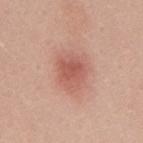workup=no biopsy performed (imaged during a skin exam)
imaging modality=~15 mm crop, total-body skin-cancer survey
location=the back
subject=male, aged approximately 30
automated lesion analysis=an average lesion color of about L≈56 a*≈26 b*≈28 (CIELAB), about 10 CIELAB-L* units darker than the surrounding skin, and a normalized lesion–skin contrast near 6.5; a border-irregularity rating of about 2.5/10 and internal color variation of about 3 on a 0–10 scale
lesion diameter=about 3.5 mm